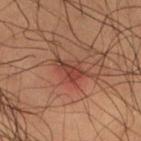Findings:
– follow-up — catalogued during a skin exam; not biopsied
– subject — male, aged 48–52
– image source — 15 mm crop, total-body photography
– image-analysis metrics — an area of roughly 4.5 mm² and a shape-asymmetry score of about 0.5 (0 = symmetric); roughly 7 lightness units darker than nearby skin; an automated nevus-likeness rating near 25 out of 100 and a lesion-detection confidence of about 100/100
– location — the right thigh
– diameter — ~3 mm (longest diameter)